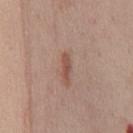– notes: no biopsy performed (imaged during a skin exam)
– acquisition: 15 mm crop, total-body photography
– illumination: white-light
– TBP lesion metrics: a footprint of about 2 mm² and an outline eccentricity of about 0.95 (0 = round, 1 = elongated); internal color variation of about 0 on a 0–10 scale and a peripheral color-asymmetry measure near 0; a nevus-likeness score of about 100/100 and a lesion-detection confidence of about 100/100
– anatomic site: the chest
– size: about 2.5 mm
– subject: female, about 45 years old